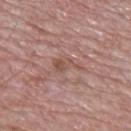notes = catalogued during a skin exam; not biopsied
subject = male, in their mid-60s
image source = ~15 mm crop, total-body skin-cancer survey
location = the back
lighting = white-light